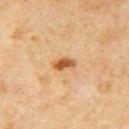  biopsy_status: not biopsied; imaged during a skin examination
  patient:
    sex: male
    age_approx: 50
  image:
    source: total-body photography crop
    field_of_view_mm: 15
  site: arm